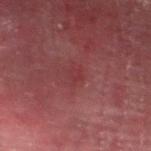The lesion was photographed on a routine skin check and not biopsied; there is no pathology result.
On the left thigh.
A male patient, approximately 55 years of age.
The tile uses cross-polarized illumination.
Approximately 2 mm at its widest.
Cropped from a total-body skin-imaging series; the visible field is about 15 mm.